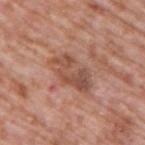Assessment:
Part of a total-body skin-imaging series; this lesion was reviewed on a skin check and was not flagged for biopsy.
Image and clinical context:
Approximately 5.5 mm at its widest. A male subject in their 70s. Imaged with white-light lighting. A region of skin cropped from a whole-body photographic capture, roughly 15 mm wide. The lesion is on the upper back.